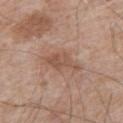Imaged during a routine full-body skin examination; the lesion was not biopsied and no histopathology is available. The subject is a male approximately 65 years of age. Cropped from a total-body skin-imaging series; the visible field is about 15 mm. Located on the left upper arm.